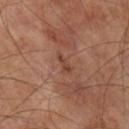biopsy_status: not biopsied; imaged during a skin examination
lighting: cross-polarized
site: right lower leg
image:
  source: total-body photography crop
  field_of_view_mm: 15
lesion_size:
  long_diameter_mm_approx: 2.5
patient:
  sex: male
  age_approx: 70